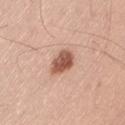  lesion_size:
    long_diameter_mm_approx: 3.5
  automated_metrics:
    area_mm2_approx: 7.0
    eccentricity: 0.7
    shape_asymmetry: 0.2
    cielab_L: 55
    cielab_a: 23
    cielab_b: 30
    vs_skin_darker_L: 16.0
    vs_skin_contrast_norm: 10.0
    border_irregularity_0_10: 2.0
    color_variation_0_10: 4.0
    peripheral_color_asymmetry: 1.5
    nevus_likeness_0_100: 90
    lesion_detection_confidence_0_100: 100
  site: left thigh
  image:
    source: total-body photography crop
    field_of_view_mm: 15
  patient:
    sex: male
    age_approx: 40
  lighting: white-light